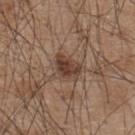{
  "biopsy_status": "not biopsied; imaged during a skin examination",
  "patient": {
    "sex": "male",
    "age_approx": 45
  },
  "lesion_size": {
    "long_diameter_mm_approx": 3.5
  },
  "site": "upper back",
  "automated_metrics": {
    "cielab_L": 40,
    "cielab_a": 19,
    "cielab_b": 25,
    "vs_skin_darker_L": 11.0,
    "vs_skin_contrast_norm": 9.0,
    "border_irregularity_0_10": 3.0,
    "peripheral_color_asymmetry": 1.5,
    "lesion_detection_confidence_0_100": 100
  },
  "image": {
    "source": "total-body photography crop",
    "field_of_view_mm": 15
  }
}